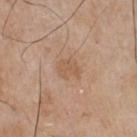Findings:
– workup — total-body-photography surveillance lesion; no biopsy
– subject — male, approximately 65 years of age
– lesion size — ≈3 mm
– TBP lesion metrics — an average lesion color of about L≈57 a*≈18 b*≈32 (CIELAB), roughly 6 lightness units darker than nearby skin, and a lesion-to-skin contrast of about 5 (normalized; higher = more distinct); a within-lesion color-variation index near 2/10 and a peripheral color-asymmetry measure near 0.5; a classifier nevus-likeness of about 0/100
– body site — the chest
– image source — 15 mm crop, total-body photography
– tile lighting — white-light illumination